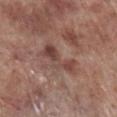Clinical impression:
Captured during whole-body skin photography for melanoma surveillance; the lesion was not biopsied.
Acquisition and patient details:
From the right lower leg. The lesion's longest dimension is about 5 mm. Cropped from a whole-body photographic skin survey; the tile spans about 15 mm. A male subject, approximately 70 years of age. The tile uses white-light illumination.